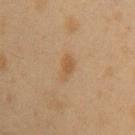Captured during whole-body skin photography for melanoma surveillance; the lesion was not biopsied. A 15 mm close-up tile from a total-body photography series done for melanoma screening. The lesion-visualizer software estimated a footprint of about 4.5 mm² and an outline eccentricity of about 0.8 (0 = round, 1 = elongated). And it measured a mean CIELAB color near L≈44 a*≈15 b*≈30, a lesion–skin lightness drop of about 6, and a normalized border contrast of about 5.5. The tile uses cross-polarized illumination. Approximately 3 mm at its widest. On the arm. A female subject roughly 40 years of age.